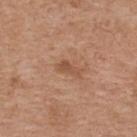Imaged during a routine full-body skin examination; the lesion was not biopsied and no histopathology is available. The recorded lesion diameter is about 3 mm. From the upper back. A female patient about 40 years old. A 15 mm close-up extracted from a 3D total-body photography capture.